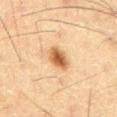Q: Was a biopsy performed?
A: no biopsy performed (imaged during a skin exam)
Q: How large is the lesion?
A: ≈3 mm
Q: How was this image acquired?
A: ~15 mm crop, total-body skin-cancer survey
Q: Automated lesion metrics?
A: an automated nevus-likeness rating near 100 out of 100 and lesion-presence confidence of about 100/100
Q: Who is the patient?
A: male, aged around 60